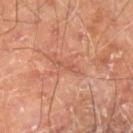Part of a total-body skin-imaging series; this lesion was reviewed on a skin check and was not flagged for biopsy. The tile uses cross-polarized illumination. A male patient aged 68–72. Cropped from a whole-body photographic skin survey; the tile spans about 15 mm. Located on the right lower leg. Approximately 3.5 mm at its widest.